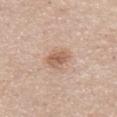Imaged during a routine full-body skin examination; the lesion was not biopsied and no histopathology is available.